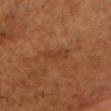| feature | finding |
|---|---|
| automated lesion analysis | a footprint of about 5 mm², an outline eccentricity of about 0.9 (0 = round, 1 = elongated), and two-axis asymmetry of about 0.3; an average lesion color of about L≈39 a*≈24 b*≈33 (CIELAB), about 5 CIELAB-L* units darker than the surrounding skin, and a normalized border contrast of about 4.5; a border-irregularity index near 3.5/10 and peripheral color asymmetry of about 0.5; a classifier nevus-likeness of about 0/100 and a detector confidence of about 95 out of 100 that the crop contains a lesion |
| site | the chest |
| diameter | about 3.5 mm |
| acquisition | ~15 mm crop, total-body skin-cancer survey |
| tile lighting | cross-polarized |
| subject | male, about 60 years old |